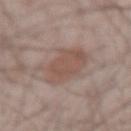Captured during whole-body skin photography for melanoma surveillance; the lesion was not biopsied. The patient is a male aged around 55. The lesion is located on the right forearm. The lesion's longest dimension is about 5.5 mm. The tile uses white-light illumination. A 15 mm close-up extracted from a 3D total-body photography capture.